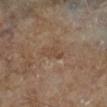Captured during whole-body skin photography for melanoma surveillance; the lesion was not biopsied.
About 3 mm across.
Captured under cross-polarized illumination.
On the leg.
A male patient roughly 85 years of age or older.
This image is a 15 mm lesion crop taken from a total-body photograph.
Automated image analysis of the tile measured an average lesion color of about L≈43 a*≈16 b*≈27 (CIELAB), a lesion–skin lightness drop of about 5, and a lesion-to-skin contrast of about 5 (normalized; higher = more distinct).